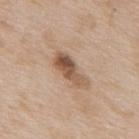This lesion was catalogued during total-body skin photography and was not selected for biopsy.
The subject is a male aged 63 to 67.
On the upper back.
This image is a 15 mm lesion crop taken from a total-body photograph.